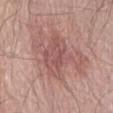Captured during whole-body skin photography for melanoma surveillance; the lesion was not biopsied. The lesion is on the abdomen. A male patient, approximately 80 years of age. A lesion tile, about 15 mm wide, cut from a 3D total-body photograph.